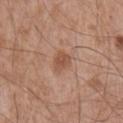The lesion was photographed on a routine skin check and not biopsied; there is no pathology result. Imaged with white-light lighting. A male patient aged approximately 65. Measured at roughly 2.5 mm in maximum diameter. From the chest. The total-body-photography lesion software estimated a lesion area of about 4.5 mm², a shape eccentricity near 0.5, and a shape-asymmetry score of about 0.25 (0 = symmetric). The software also gave a mean CIELAB color near L≈52 a*≈22 b*≈30 and about 9 CIELAB-L* units darker than the surrounding skin. This image is a 15 mm lesion crop taken from a total-body photograph.